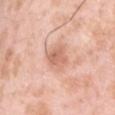Assessment:
Part of a total-body skin-imaging series; this lesion was reviewed on a skin check and was not flagged for biopsy.
Context:
This is a white-light tile. A male patient, aged approximately 35. A 15 mm close-up tile from a total-body photography series done for melanoma screening. The total-body-photography lesion software estimated a footprint of about 7.5 mm² and a symmetry-axis asymmetry near 0.25. And it measured a lesion color around L≈66 a*≈24 b*≈31 in CIELAB and a normalized lesion–skin contrast near 6.5. It also reported a border-irregularity index near 2.5/10. The analysis additionally found an automated nevus-likeness rating near 10 out of 100 and lesion-presence confidence of about 100/100. About 4 mm across. The lesion is located on the chest.